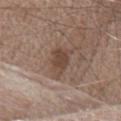{
  "biopsy_status": "not biopsied; imaged during a skin examination",
  "automated_metrics": {
    "border_irregularity_0_10": 3.0,
    "color_variation_0_10": 3.0,
    "peripheral_color_asymmetry": 1.0,
    "nevus_likeness_0_100": 5,
    "lesion_detection_confidence_0_100": 100
  },
  "patient": {
    "sex": "male",
    "age_approx": 70
  },
  "image": {
    "source": "total-body photography crop",
    "field_of_view_mm": 15
  },
  "site": "chest"
}